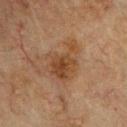Impression:
This lesion was catalogued during total-body skin photography and was not selected for biopsy.